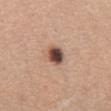| key | value |
|---|---|
| image | total-body-photography crop, ~15 mm field of view |
| patient | female, aged 53–57 |
| automated metrics | a lesion area of about 5.5 mm², a shape eccentricity near 0.45, and a symmetry-axis asymmetry near 0.2 |
| anatomic site | the abdomen |
| lesion size | ~2.5 mm (longest diameter) |
| lighting | white-light |